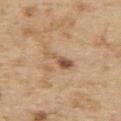  biopsy_status: not biopsied; imaged during a skin examination
  patient:
    sex: male
    age_approx: 70
  lighting: white-light
  image:
    source: total-body photography crop
    field_of_view_mm: 15
  site: back
  lesion_size:
    long_diameter_mm_approx: 4.0